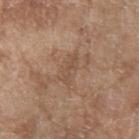Captured during whole-body skin photography for melanoma surveillance; the lesion was not biopsied. A female patient, in their mid- to late 70s. The lesion is on the right forearm. Cropped from a total-body skin-imaging series; the visible field is about 15 mm. This is a white-light tile.